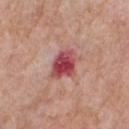Image and clinical context: The lesion is located on the chest. A male subject approximately 60 years of age. The tile uses white-light illumination. A roughly 15 mm field-of-view crop from a total-body skin photograph.